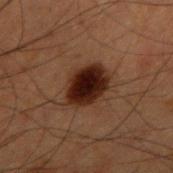workup = catalogued during a skin exam; not biopsied | anatomic site = the right upper arm | imaging modality = 15 mm crop, total-body photography | TBP lesion metrics = a footprint of about 14 mm² and a symmetry-axis asymmetry near 0.2; a mean CIELAB color near L≈18 a*≈16 b*≈19, a lesion–skin lightness drop of about 12, and a normalized lesion–skin contrast near 14.5; a nevus-likeness score of about 100/100 | patient = male, aged around 60 | size = ≈4.5 mm | illumination = cross-polarized illumination.